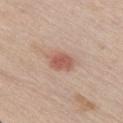Q: Was this lesion biopsied?
A: no biopsy performed (imaged during a skin exam)
Q: Automated lesion metrics?
A: an automated nevus-likeness rating near 65 out of 100 and lesion-presence confidence of about 100/100
Q: Patient demographics?
A: male, aged around 65
Q: What is the lesion's diameter?
A: ≈3 mm
Q: What kind of image is this?
A: total-body-photography crop, ~15 mm field of view
Q: What is the anatomic site?
A: the chest
Q: What lighting was used for the tile?
A: white-light illumination